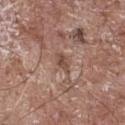This lesion was catalogued during total-body skin photography and was not selected for biopsy. A male subject, aged approximately 70. Automated image analysis of the tile measured a within-lesion color-variation index near 2/10 and peripheral color asymmetry of about 1. And it measured a nevus-likeness score of about 0/100. The lesion is on the chest. Measured at roughly 3 mm in maximum diameter. A 15 mm crop from a total-body photograph taken for skin-cancer surveillance. Imaged with white-light lighting.